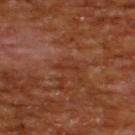workup: total-body-photography surveillance lesion; no biopsy | body site: the upper back | TBP lesion metrics: a shape eccentricity near 0.95; an average lesion color of about L≈32 a*≈24 b*≈31 (CIELAB), about 5 CIELAB-L* units darker than the surrounding skin, and a normalized border contrast of about 5.5; lesion-presence confidence of about 85/100 | patient: male, aged around 65 | lighting: cross-polarized illumination | image: total-body-photography crop, ~15 mm field of view.